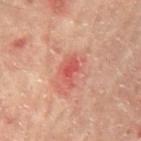Notes:
• biopsy status — total-body-photography surveillance lesion; no biopsy
• acquisition — 15 mm crop, total-body photography
• illumination — cross-polarized
• patient — male, in their mid- to late 60s
• site — the mid back
• size — ~3 mm (longest diameter)